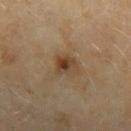biopsy_status: not biopsied; imaged during a skin examination
patient:
  sex: female
  age_approx: 60
site: left forearm
lesion_size:
  long_diameter_mm_approx: 3.0
image:
  source: total-body photography crop
  field_of_view_mm: 15
lighting: cross-polarized